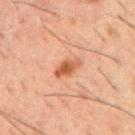– notes — catalogued during a skin exam; not biopsied